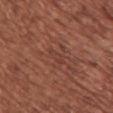The lesion was tiled from a total-body skin photograph and was not biopsied. Approximately 3 mm at its widest. The subject is a female approximately 65 years of age. This is a white-light tile. From the chest. Cropped from a whole-body photographic skin survey; the tile spans about 15 mm.